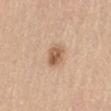Q: Is there a histopathology result?
A: total-body-photography surveillance lesion; no biopsy
Q: Illumination type?
A: white-light illumination
Q: Automated lesion metrics?
A: a within-lesion color-variation index near 5/10 and radial color variation of about 1.5; a nevus-likeness score of about 95/100 and a lesion-detection confidence of about 100/100
Q: How was this image acquired?
A: total-body-photography crop, ~15 mm field of view
Q: Lesion location?
A: the lower back
Q: What are the patient's age and sex?
A: female, about 65 years old
Q: Lesion size?
A: about 2.5 mm